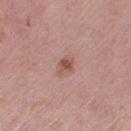No biopsy was performed on this lesion — it was imaged during a full skin examination and was not determined to be concerning.
A lesion tile, about 15 mm wide, cut from a 3D total-body photograph.
On the right thigh.
Measured at roughly 2.5 mm in maximum diameter.
The total-body-photography lesion software estimated an average lesion color of about L≈53 a*≈22 b*≈25 (CIELAB), roughly 9 lightness units darker than nearby skin, and a normalized lesion–skin contrast near 7. And it measured border irregularity of about 2.5 on a 0–10 scale, a within-lesion color-variation index near 3/10, and peripheral color asymmetry of about 1.
A female subject aged around 65.